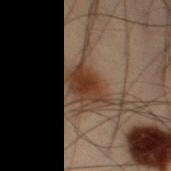biopsy status: imaged on a skin check; not biopsied | subject: male, aged approximately 55 | imaging modality: total-body-photography crop, ~15 mm field of view | site: the leg | illumination: cross-polarized.